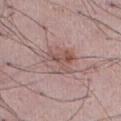follow-up: imaged on a skin check; not biopsied
anatomic site: the abdomen
automated lesion analysis: a lesion area of about 11 mm², an eccentricity of roughly 0.55, and two-axis asymmetry of about 0.2; a lesion color around L≈54 a*≈19 b*≈22 in CIELAB and a normalized lesion–skin contrast near 6; a border-irregularity index near 3/10 and a peripheral color-asymmetry measure near 3; a nevus-likeness score of about 25/100
patient: male, aged around 55
imaging modality: 15 mm crop, total-body photography
diameter: ≈4 mm